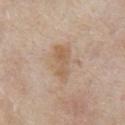Recorded during total-body skin imaging; not selected for excision or biopsy.
A close-up tile cropped from a whole-body skin photograph, about 15 mm across.
A male subject approximately 65 years of age.
The lesion's longest dimension is about 4.5 mm.
On the front of the torso.
Automated image analysis of the tile measured a lesion color around L≈59 a*≈16 b*≈32 in CIELAB, roughly 8 lightness units darker than nearby skin, and a normalized border contrast of about 6.5. The analysis additionally found a border-irregularity index near 3.5/10 and a within-lesion color-variation index near 2.5/10.